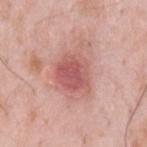Clinical impression:
Imaged during a routine full-body skin examination; the lesion was not biopsied and no histopathology is available.
Acquisition and patient details:
Cropped from a whole-body photographic skin survey; the tile spans about 15 mm. On the upper back. The patient is a male aged approximately 35. This is a white-light tile.